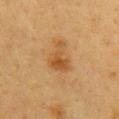Assessment: This lesion was catalogued during total-body skin photography and was not selected for biopsy. Background: A roughly 15 mm field-of-view crop from a total-body skin photograph. Measured at roughly 4 mm in maximum diameter. A female patient in their 40s. The lesion is located on the chest. The tile uses cross-polarized illumination.